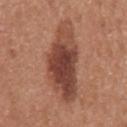Impression: This lesion was catalogued during total-body skin photography and was not selected for biopsy. Image and clinical context: Captured under white-light illumination. A female subject aged 48 to 52. A 15 mm close-up tile from a total-body photography series done for melanoma screening. The lesion is on the left upper arm. The lesion's longest dimension is about 8 mm.